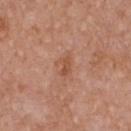Assessment: Captured during whole-body skin photography for melanoma surveillance; the lesion was not biopsied. Context: A 15 mm close-up extracted from a 3D total-body photography capture. The subject is a female aged 38–42. From the chest.